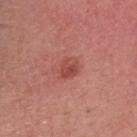The lesion was tiled from a total-body skin photograph and was not biopsied. The tile uses white-light illumination. The lesion is on the head or neck. A male patient, aged around 30. A 15 mm crop from a total-body photograph taken for skin-cancer surveillance.